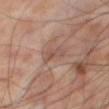Q: Was this lesion biopsied?
A: imaged on a skin check; not biopsied
Q: Lesion location?
A: the right thigh
Q: Illumination type?
A: cross-polarized
Q: Lesion size?
A: ~2.5 mm (longest diameter)
Q: What are the patient's age and sex?
A: male, roughly 60 years of age
Q: What kind of image is this?
A: ~15 mm crop, total-body skin-cancer survey